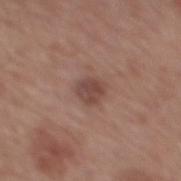biopsy status = imaged on a skin check; not biopsied | acquisition = 15 mm crop, total-body photography | subject = male, about 75 years old | location = the mid back | size = ≈2.5 mm.